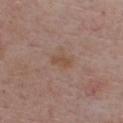acquisition=total-body-photography crop, ~15 mm field of view; subject=male, about 75 years old; body site=the chest; diameter=≈2.5 mm; illumination=white-light illumination.